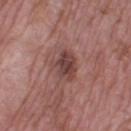* workup: imaged on a skin check; not biopsied
* subject: male, about 55 years old
* acquisition: total-body-photography crop, ~15 mm field of view
* site: the right lower leg